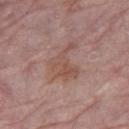<record>
  <biopsy_status>not biopsied; imaged during a skin examination</biopsy_status>
  <lesion_size>
    <long_diameter_mm_approx>5.0</long_diameter_mm_approx>
  </lesion_size>
  <site>left thigh</site>
  <patient>
    <sex>female</sex>
    <age_approx>65</age_approx>
  </patient>
  <lighting>white-light</lighting>
  <image>
    <source>total-body photography crop</source>
    <field_of_view_mm>15</field_of_view_mm>
  </image>
</record>